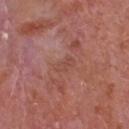biopsy status — imaged on a skin check; not biopsied | image source — ~15 mm tile from a whole-body skin photo | site — the front of the torso | patient — male, roughly 65 years of age.